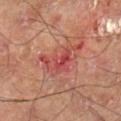{"biopsy_status": "not biopsied; imaged during a skin examination", "site": "right lower leg", "lesion_size": {"long_diameter_mm_approx": 5.5}, "image": {"source": "total-body photography crop", "field_of_view_mm": 15}, "lighting": "cross-polarized"}